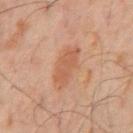- location — the abdomen
- automated lesion analysis — an automated nevus-likeness rating near 25 out of 100 and a lesion-detection confidence of about 100/100
- lighting — cross-polarized
- imaging modality — ~15 mm tile from a whole-body skin photo
- subject — male, aged 58 to 62
- diameter — ≈4.5 mm